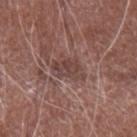A 15 mm close-up extracted from a 3D total-body photography capture. Located on the right forearm. This is a white-light tile. A male patient in their mid- to late 60s.